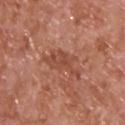Recorded during total-body skin imaging; not selected for excision or biopsy. This image is a 15 mm lesion crop taken from a total-body photograph. Located on the chest. A male subject aged around 65. The tile uses white-light illumination.